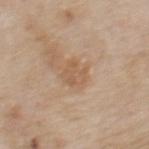workup: total-body-photography surveillance lesion; no biopsy | subject: male, aged around 85 or older | lesion size: ≈3 mm | automated lesion analysis: an average lesion color of about L≈57 a*≈18 b*≈33 (CIELAB), a lesion–skin lightness drop of about 7, and a lesion-to-skin contrast of about 5.5 (normalized; higher = more distinct); a border-irregularity index near 3/10 and radial color variation of about 1; an automated nevus-likeness rating near 0 out of 100 and a detector confidence of about 100 out of 100 that the crop contains a lesion | imaging modality: ~15 mm tile from a whole-body skin photo | illumination: white-light | anatomic site: the upper back.